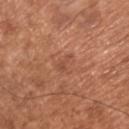workup=no biopsy performed (imaged during a skin exam) | subject=male, in their mid- to late 50s | image source=15 mm crop, total-body photography | site=the chest | lesion diameter=≈3 mm | tile lighting=white-light.